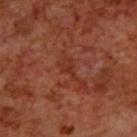| field | value |
|---|---|
| biopsy status | catalogued during a skin exam; not biopsied |
| body site | the upper back |
| diameter | about 3 mm |
| lighting | cross-polarized |
| acquisition | ~15 mm crop, total-body skin-cancer survey |
| subject | male, aged 68–72 |